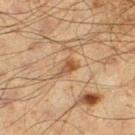The lesion was photographed on a routine skin check and not biopsied; there is no pathology result.
Approximately 3 mm at its widest.
From the left thigh.
The subject is a male aged 58–62.
A close-up tile cropped from a whole-body skin photograph, about 15 mm across.
The lesion-visualizer software estimated an average lesion color of about L≈44 a*≈17 b*≈31 (CIELAB), a lesion–skin lightness drop of about 9, and a normalized lesion–skin contrast near 7. The analysis additionally found peripheral color asymmetry of about 0.5. It also reported a classifier nevus-likeness of about 35/100.